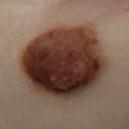* workup — total-body-photography surveillance lesion; no biopsy
* patient — male, aged 48 to 52
* automated metrics — an average lesion color of about L≈24 a*≈16 b*≈19 (CIELAB), about 18 CIELAB-L* units darker than the surrounding skin, and a lesion-to-skin contrast of about 17.5 (normalized; higher = more distinct); a within-lesion color-variation index near 9.5/10
* image — ~15 mm tile from a whole-body skin photo
* body site — the left leg
* tile lighting — cross-polarized